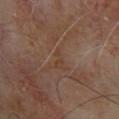Findings:
• follow-up · no biopsy performed (imaged during a skin exam)
• anatomic site · the upper back
• patient · male, roughly 65 years of age
• image · 15 mm crop, total-body photography
• lighting · cross-polarized
• automated lesion analysis · a shape eccentricity near 0.9 and two-axis asymmetry of about 0.4; a border-irregularity index near 4/10 and a peripheral color-asymmetry measure near 0
• lesion size · ~2.5 mm (longest diameter)